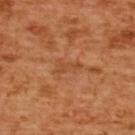biopsy_status: not biopsied; imaged during a skin examination
image:
  source: total-body photography crop
  field_of_view_mm: 15
lighting: cross-polarized
patient:
  sex: female
  age_approx: 55
lesion_size:
  long_diameter_mm_approx: 3.5
site: upper back